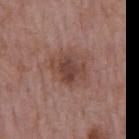Notes:
• biopsy status · catalogued during a skin exam; not biopsied
• image source · ~15 mm crop, total-body skin-cancer survey
• illumination · white-light illumination
• subject · male, aged approximately 75
• site · the mid back
• automated metrics · a lesion color around L≈43 a*≈20 b*≈24 in CIELAB and a lesion–skin lightness drop of about 9; a classifier nevus-likeness of about 10/100
• lesion size · ~4 mm (longest diameter)